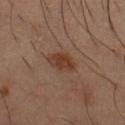Assessment: This lesion was catalogued during total-body skin photography and was not selected for biopsy. Acquisition and patient details: The subject is a male roughly 35 years of age. On the chest. A 15 mm close-up tile from a total-body photography series done for melanoma screening.